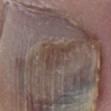Imaged during a routine full-body skin examination; the lesion was not biopsied and no histopathology is available. Captured under white-light illumination. The lesion is located on the left lower leg. A male subject aged 73–77. Longest diameter approximately 4.5 mm. A 15 mm close-up extracted from a 3D total-body photography capture.